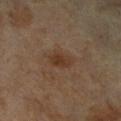Imaged during a routine full-body skin examination; the lesion was not biopsied and no histopathology is available. The lesion is on the left leg. A close-up tile cropped from a whole-body skin photograph, about 15 mm across. Automated tile analysis of the lesion measured roughly 7 lightness units darker than nearby skin and a normalized border contrast of about 7.5. This is a cross-polarized tile. The subject is a female aged approximately 65.